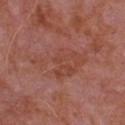{
  "biopsy_status": "not biopsied; imaged during a skin examination",
  "image": {
    "source": "total-body photography crop",
    "field_of_view_mm": 15
  },
  "site": "front of the torso",
  "lighting": "white-light",
  "automated_metrics": {
    "area_mm2_approx": 15.0,
    "eccentricity": 0.85,
    "shape_asymmetry": 0.45
  },
  "lesion_size": {
    "long_diameter_mm_approx": 7.0
  },
  "patient": {
    "sex": "male",
    "age_approx": 65
  }
}